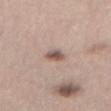  biopsy_status: not biopsied; imaged during a skin examination
  site: abdomen
  lighting: white-light
  image:
    source: total-body photography crop
    field_of_view_mm: 15
  patient:
    sex: female
    age_approx: 40